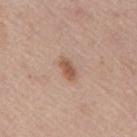Clinical impression:
No biopsy was performed on this lesion — it was imaged during a full skin examination and was not determined to be concerning.
Acquisition and patient details:
Cropped from a whole-body photographic skin survey; the tile spans about 15 mm. About 3 mm across. A female patient about 65 years old. Captured under white-light illumination. An algorithmic analysis of the crop reported a lesion color around L≈55 a*≈20 b*≈30 in CIELAB and a lesion-to-skin contrast of about 8 (normalized; higher = more distinct). The software also gave a border-irregularity index near 2.5/10, a within-lesion color-variation index near 2/10, and radial color variation of about 0.5. It also reported a nevus-likeness score of about 90/100. The lesion is located on the back.